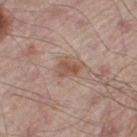biopsy status: catalogued during a skin exam; not biopsied
automated lesion analysis: an area of roughly 4 mm² and a symmetry-axis asymmetry near 0.3; a detector confidence of about 100 out of 100 that the crop contains a lesion
tile lighting: white-light
site: the right thigh
lesion size: ≈3 mm
subject: male, approximately 55 years of age
image: 15 mm crop, total-body photography